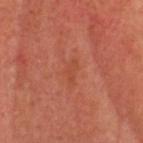Assessment: This lesion was catalogued during total-body skin photography and was not selected for biopsy. Background: Automated tile analysis of the lesion measured a mean CIELAB color near L≈46 a*≈30 b*≈34 and a lesion-to-skin contrast of about 4.5 (normalized; higher = more distinct). Longest diameter approximately 2.5 mm. A roughly 15 mm field-of-view crop from a total-body skin photograph. The lesion is on the head or neck. The subject is a male aged 58–62. The tile uses cross-polarized illumination.